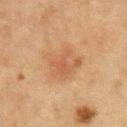Part of a total-body skin-imaging series; this lesion was reviewed on a skin check and was not flagged for biopsy. The recorded lesion diameter is about 5.5 mm. The lesion is on the chest. A lesion tile, about 15 mm wide, cut from a 3D total-body photograph. A male patient, aged 63–67.